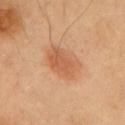Part of a total-body skin-imaging series; this lesion was reviewed on a skin check and was not flagged for biopsy. From the chest. A 15 mm crop from a total-body photograph taken for skin-cancer surveillance.